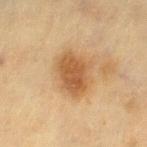Assessment:
This lesion was catalogued during total-body skin photography and was not selected for biopsy.
Image and clinical context:
Imaged with cross-polarized lighting. Cropped from a total-body skin-imaging series; the visible field is about 15 mm. Located on the left thigh. A female patient, approximately 55 years of age.